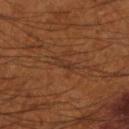Captured during whole-body skin photography for melanoma surveillance; the lesion was not biopsied. The lesion is on the right forearm. The tile uses cross-polarized illumination. The subject is a male approximately 55 years of age. A roughly 15 mm field-of-view crop from a total-body skin photograph. Approximately 2.5 mm at its widest.